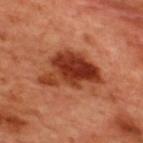Findings:
• biopsy status · catalogued during a skin exam; not biopsied
• lesion diameter · ≈7 mm
• location · the upper back
• TBP lesion metrics · an area of roughly 22 mm², a shape eccentricity near 0.75, and two-axis asymmetry of about 0.4
• subject · male, aged around 50
• image source · 15 mm crop, total-body photography